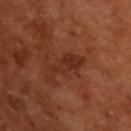Background: This is a cross-polarized tile. A roughly 15 mm field-of-view crop from a total-body skin photograph. A male subject approximately 65 years of age. About 5.5 mm across.